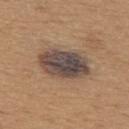<case>
  <biopsy_status>not biopsied; imaged during a skin examination</biopsy_status>
  <lighting>white-light</lighting>
  <image>
    <source>total-body photography crop</source>
    <field_of_view_mm>15</field_of_view_mm>
  </image>
  <patient>
    <sex>male</sex>
    <age_approx>65</age_approx>
  </patient>
  <site>upper back</site>
  <lesion_size>
    <long_diameter_mm_approx>6.5</long_diameter_mm_approx>
  </lesion_size>
</case>